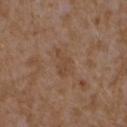Assessment: Captured during whole-body skin photography for melanoma surveillance; the lesion was not biopsied. Acquisition and patient details: The lesion is on the right forearm. A close-up tile cropped from a whole-body skin photograph, about 15 mm across. Captured under white-light illumination. Measured at roughly 3.5 mm in maximum diameter. A female patient, in their mid- to late 30s.